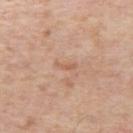Part of a total-body skin-imaging series; this lesion was reviewed on a skin check and was not flagged for biopsy. Located on the left upper arm. This image is a 15 mm lesion crop taken from a total-body photograph. Measured at roughly 2.5 mm in maximum diameter. This is a white-light tile. A male subject, approximately 65 years of age.